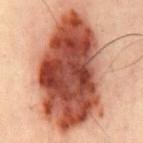lesion diameter — ≈14.5 mm | acquisition — ~15 mm tile from a whole-body skin photo | body site — the chest | patient — male, approximately 50 years of age.